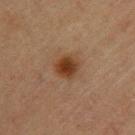{
  "biopsy_status": "not biopsied; imaged during a skin examination",
  "patient": {
    "sex": "female",
    "age_approx": 65
  },
  "automated_metrics": {
    "area_mm2_approx": 6.5,
    "eccentricity": 0.4,
    "shape_asymmetry": 0.2,
    "border_irregularity_0_10": 1.5,
    "color_variation_0_10": 4.0,
    "peripheral_color_asymmetry": 1.0,
    "nevus_likeness_0_100": 100,
    "lesion_detection_confidence_0_100": 100
  },
  "lesion_size": {
    "long_diameter_mm_approx": 3.0
  },
  "image": {
    "source": "total-body photography crop",
    "field_of_view_mm": 15
  },
  "site": "upper back",
  "lighting": "cross-polarized"
}